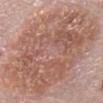Part of a total-body skin-imaging series; this lesion was reviewed on a skin check and was not flagged for biopsy. From the front of the torso. An algorithmic analysis of the crop reported a footprint of about 80 mm², an outline eccentricity of about 0.75 (0 = round, 1 = elongated), and two-axis asymmetry of about 0.25. This is a white-light tile. The recorded lesion diameter is about 13 mm. A male subject aged 78–82. This image is a 15 mm lesion crop taken from a total-body photograph.